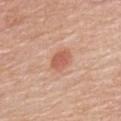The lesion was tiled from a total-body skin photograph and was not biopsied. A region of skin cropped from a whole-body photographic capture, roughly 15 mm wide. Imaged with white-light lighting. The recorded lesion diameter is about 3 mm. The lesion is located on the upper back. The patient is a female aged 63–67.